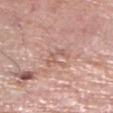Assessment:
Captured during whole-body skin photography for melanoma surveillance; the lesion was not biopsied.
Image and clinical context:
The subject is a female aged 68–72. Cropped from a whole-body photographic skin survey; the tile spans about 15 mm. This is a white-light tile. The lesion's longest dimension is about 3 mm. The lesion is on the right lower leg.